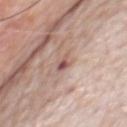Context:
The patient is a male approximately 80 years of age. Cropped from a whole-body photographic skin survey; the tile spans about 15 mm. The tile uses white-light illumination. The lesion's longest dimension is about 3.5 mm. Automated image analysis of the tile measured a mean CIELAB color near L≈57 a*≈19 b*≈23. It also reported a nevus-likeness score of about 0/100. The lesion is located on the chest.